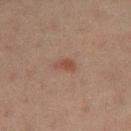Findings:
* follow-up · total-body-photography surveillance lesion; no biopsy
* automated lesion analysis · a footprint of about 3 mm² and a shape-asymmetry score of about 0.35 (0 = symmetric); an average lesion color of about L≈41 a*≈19 b*≈25 (CIELAB), roughly 7 lightness units darker than nearby skin, and a normalized lesion–skin contrast near 6.5; a border-irregularity rating of about 3.5/10, a color-variation rating of about 0.5/10, and a peripheral color-asymmetry measure near 0.5; a nevus-likeness score of about 65/100
* lighting · cross-polarized illumination
* location · the left thigh
* imaging modality · ~15 mm crop, total-body skin-cancer survey
* size · ≈2.5 mm
* patient · female, in their 20s